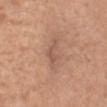Impression: The lesion was photographed on a routine skin check and not biopsied; there is no pathology result.